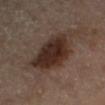The lesion was photographed on a routine skin check and not biopsied; there is no pathology result. A female patient, aged around 65. Automated image analysis of the tile measured a lesion area of about 24 mm² and a shape-asymmetry score of about 0.25 (0 = symmetric). It also reported roughly 11 lightness units darker than nearby skin and a normalized border contrast of about 11. The analysis additionally found a classifier nevus-likeness of about 80/100 and a detector confidence of about 100 out of 100 that the crop contains a lesion. Captured under cross-polarized illumination. About 6 mm across. A region of skin cropped from a whole-body photographic capture, roughly 15 mm wide. On the right upper arm.